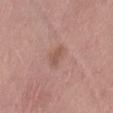No biopsy was performed on this lesion — it was imaged during a full skin examination and was not determined to be concerning. A female subject, aged 53 to 57. About 3 mm across. This image is a 15 mm lesion crop taken from a total-body photograph. On the leg. The total-body-photography lesion software estimated a lesion color around L≈54 a*≈21 b*≈26 in CIELAB and a lesion-to-skin contrast of about 5.5 (normalized; higher = more distinct). And it measured border irregularity of about 3 on a 0–10 scale and peripheral color asymmetry of about 0.5. The software also gave an automated nevus-likeness rating near 0 out of 100.